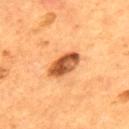Clinical impression: This lesion was catalogued during total-body skin photography and was not selected for biopsy. Image and clinical context: This is a cross-polarized tile. A male patient aged approximately 55. On the upper back. Approximately 4.5 mm at its widest. This image is a 15 mm lesion crop taken from a total-body photograph.